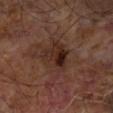Clinical impression:
The lesion was photographed on a routine skin check and not biopsied; there is no pathology result.
Image and clinical context:
Cropped from a total-body skin-imaging series; the visible field is about 15 mm. This is a cross-polarized tile. A male subject aged approximately 65. The total-body-photography lesion software estimated an area of roughly 10 mm², an outline eccentricity of about 0.65 (0 = round, 1 = elongated), and a symmetry-axis asymmetry near 0.3. The analysis additionally found an average lesion color of about L≈25 a*≈17 b*≈22 (CIELAB) and a lesion-to-skin contrast of about 9 (normalized; higher = more distinct). And it measured a border-irregularity index near 3.5/10, a within-lesion color-variation index near 8/10, and peripheral color asymmetry of about 3. It also reported lesion-presence confidence of about 100/100. The lesion's longest dimension is about 4.5 mm. The lesion is on the right forearm.